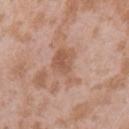{"biopsy_status": "not biopsied; imaged during a skin examination", "lighting": "white-light", "patient": {"sex": "female", "age_approx": 25}, "image": {"source": "total-body photography crop", "field_of_view_mm": 15}, "lesion_size": {"long_diameter_mm_approx": 5.0}, "site": "left upper arm"}